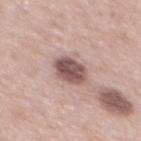Q: Is there a histopathology result?
A: no biopsy performed (imaged during a skin exam)
Q: How was the tile lit?
A: white-light
Q: What is the lesion's diameter?
A: about 3.5 mm
Q: Lesion location?
A: the mid back
Q: How was this image acquired?
A: ~15 mm crop, total-body skin-cancer survey
Q: Who is the patient?
A: male, about 65 years old